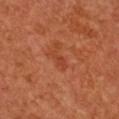{"biopsy_status": "not biopsied; imaged during a skin examination", "site": "chest", "patient": {"sex": "female", "age_approx": 65}, "image": {"source": "total-body photography crop", "field_of_view_mm": 15}, "lighting": "cross-polarized", "lesion_size": {"long_diameter_mm_approx": 3.0}}